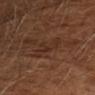Captured during whole-body skin photography for melanoma surveillance; the lesion was not biopsied. A male subject aged 63–67. A 15 mm crop from a total-body photograph taken for skin-cancer surveillance.